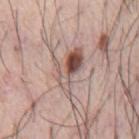Impression:
Captured during whole-body skin photography for melanoma surveillance; the lesion was not biopsied.
Background:
Automated tile analysis of the lesion measured a lesion area of about 10 mm². A region of skin cropped from a whole-body photographic capture, roughly 15 mm wide. Imaged with white-light lighting. On the chest. The subject is a male aged around 75. Longest diameter approximately 5.5 mm.